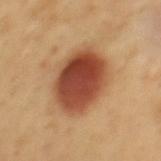Imaged during a routine full-body skin examination; the lesion was not biopsied and no histopathology is available. Automated image analysis of the tile measured a lesion area of about 27 mm², an outline eccentricity of about 0.6 (0 = round, 1 = elongated), and a shape-asymmetry score of about 0.1 (0 = symmetric). The software also gave a lesion color around L≈47 a*≈26 b*≈34 in CIELAB, a lesion–skin lightness drop of about 17, and a lesion-to-skin contrast of about 12 (normalized; higher = more distinct). It also reported a color-variation rating of about 6.5/10 and radial color variation of about 2. The software also gave a nevus-likeness score of about 100/100 and lesion-presence confidence of about 100/100. Imaged with cross-polarized lighting. About 6.5 mm across. A 15 mm close-up tile from a total-body photography series done for melanoma screening. A male patient, roughly 50 years of age. The lesion is located on the mid back.